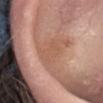Assessment: This lesion was catalogued during total-body skin photography and was not selected for biopsy. Clinical summary: A close-up tile cropped from a whole-body skin photograph, about 15 mm across. On the head or neck. A female subject aged approximately 25. About 3.5 mm across. This is a white-light tile.